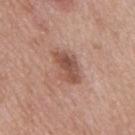follow-up=no biopsy performed (imaged during a skin exam); lesion diameter=≈4.5 mm; illumination=white-light; subject=male, in their mid-50s; site=the back; image=15 mm crop, total-body photography.